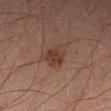workup: total-body-photography surveillance lesion; no biopsy
lighting: cross-polarized illumination
body site: the leg
patient: male, about 30 years old
automated lesion analysis: a mean CIELAB color near L≈30 a*≈16 b*≈22, roughly 7 lightness units darker than nearby skin, and a lesion-to-skin contrast of about 7.5 (normalized; higher = more distinct); lesion-presence confidence of about 100/100
acquisition: 15 mm crop, total-body photography